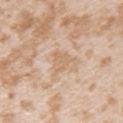– biopsy status · imaged on a skin check; not biopsied
– image-analysis metrics · a lesion area of about 4.5 mm², a shape eccentricity near 0.55, and a symmetry-axis asymmetry near 0.45; a border-irregularity index near 5/10, internal color variation of about 1.5 on a 0–10 scale, and a peripheral color-asymmetry measure near 0.5; a classifier nevus-likeness of about 0/100 and a lesion-detection confidence of about 100/100
– subject · female, in their mid-20s
– body site · the left upper arm
– image source · ~15 mm crop, total-body skin-cancer survey
– tile lighting · white-light